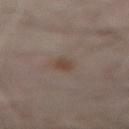Imaged during a routine full-body skin examination; the lesion was not biopsied and no histopathology is available. A male subject, about 50 years old. This is a cross-polarized tile. The lesion is on the abdomen. A region of skin cropped from a whole-body photographic capture, roughly 15 mm wide.